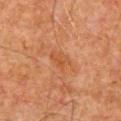Findings:
– workup — imaged on a skin check; not biopsied
– imaging modality — ~15 mm crop, total-body skin-cancer survey
– patient — male, aged approximately 80
– site — the front of the torso